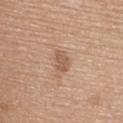Background:
A close-up tile cropped from a whole-body skin photograph, about 15 mm across. On the upper back. Longest diameter approximately 3 mm. A female subject, roughly 65 years of age.